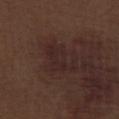Impression:
This lesion was catalogued during total-body skin photography and was not selected for biopsy.
Context:
The total-body-photography lesion software estimated an area of roughly 9.5 mm² and two-axis asymmetry of about 0.45. Measured at roughly 5 mm in maximum diameter. Cropped from a whole-body photographic skin survey; the tile spans about 15 mm. A male patient aged around 70. From the leg. Imaged with white-light lighting.